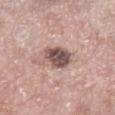This lesion was catalogued during total-body skin photography and was not selected for biopsy. A male patient, aged approximately 80. The lesion is on the left thigh. A 15 mm crop from a total-body photograph taken for skin-cancer surveillance. Longest diameter approximately 3.5 mm. Imaged with white-light lighting.